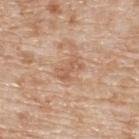Part of a total-body skin-imaging series; this lesion was reviewed on a skin check and was not flagged for biopsy.
On the upper back.
The patient is a male aged 78 to 82.
About 3.5 mm across.
A lesion tile, about 15 mm wide, cut from a 3D total-body photograph.
This is a white-light tile.